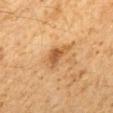The lesion is located on the mid back. The patient is a male approximately 60 years of age. This is a cross-polarized tile. A roughly 15 mm field-of-view crop from a total-body skin photograph.